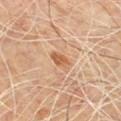Impression:
No biopsy was performed on this lesion — it was imaged during a full skin examination and was not determined to be concerning.
Clinical summary:
Measured at roughly 3 mm in maximum diameter. A 15 mm close-up tile from a total-body photography series done for melanoma screening. From the chest. The patient is a male roughly 70 years of age. Imaged with cross-polarized lighting. Automated image analysis of the tile measured an area of roughly 3.5 mm² and an eccentricity of roughly 0.9. And it measured a lesion color around L≈58 a*≈22 b*≈37 in CIELAB, about 10 CIELAB-L* units darker than the surrounding skin, and a lesion-to-skin contrast of about 7.5 (normalized; higher = more distinct). And it measured a border-irregularity rating of about 2.5/10, a color-variation rating of about 2/10, and peripheral color asymmetry of about 0.5.